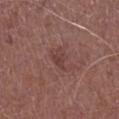Captured during whole-body skin photography for melanoma surveillance; the lesion was not biopsied. A male patient roughly 75 years of age. Located on the leg. A close-up tile cropped from a whole-body skin photograph, about 15 mm across.